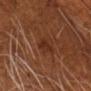follow-up=catalogued during a skin exam; not biopsied
diameter=≈3 mm
body site=the head or neck
lighting=cross-polarized illumination
image source=~15 mm tile from a whole-body skin photo
TBP lesion metrics=an area of roughly 3 mm², a shape eccentricity near 0.8, and a symmetry-axis asymmetry near 0.45; a border-irregularity rating of about 5/10, internal color variation of about 0.5 on a 0–10 scale, and a peripheral color-asymmetry measure near 0; a classifier nevus-likeness of about 15/100 and lesion-presence confidence of about 100/100
patient=male, about 60 years old